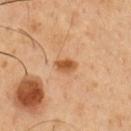Assessment: Captured during whole-body skin photography for melanoma surveillance; the lesion was not biopsied. Image and clinical context: Captured under cross-polarized illumination. A male subject, aged around 50. The lesion's longest dimension is about 2.5 mm. From the chest. A 15 mm close-up tile from a total-body photography series done for melanoma screening. The total-body-photography lesion software estimated an outline eccentricity of about 0.75 (0 = round, 1 = elongated) and two-axis asymmetry of about 0.3. The software also gave a lesion color around L≈55 a*≈24 b*≈40 in CIELAB, about 12 CIELAB-L* units darker than the surrounding skin, and a normalized border contrast of about 9. The software also gave a nevus-likeness score of about 90/100 and a lesion-detection confidence of about 100/100.